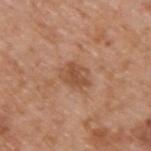workup: catalogued during a skin exam; not biopsied
patient: male, aged around 65
image source: total-body-photography crop, ~15 mm field of view
lighting: white-light illumination
anatomic site: the upper back
size: ≈3.5 mm